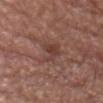Q: Is there a histopathology result?
A: imaged on a skin check; not biopsied
Q: Lesion location?
A: the chest
Q: Illumination type?
A: white-light illumination
Q: What are the patient's age and sex?
A: male, approximately 80 years of age
Q: What kind of image is this?
A: ~15 mm tile from a whole-body skin photo
Q: Automated lesion metrics?
A: a mean CIELAB color near L≈40 a*≈21 b*≈24, about 8 CIELAB-L* units darker than the surrounding skin, and a normalized border contrast of about 6.5; internal color variation of about 3 on a 0–10 scale and peripheral color asymmetry of about 1